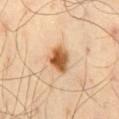| feature | finding |
|---|---|
| workup | imaged on a skin check; not biopsied |